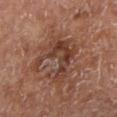Captured during whole-body skin photography for melanoma surveillance; the lesion was not biopsied. On the left lower leg. Imaged with cross-polarized lighting. A male patient, about 65 years old. Cropped from a whole-body photographic skin survey; the tile spans about 15 mm. About 5.5 mm across.